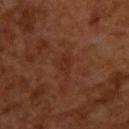Part of a total-body skin-imaging series; this lesion was reviewed on a skin check and was not flagged for biopsy. A roughly 15 mm field-of-view crop from a total-body skin photograph. This is a cross-polarized tile. A male patient, aged approximately 65. The lesion-visualizer software estimated an area of roughly 4 mm² and a shape-asymmetry score of about 0.3 (0 = symmetric). The software also gave an average lesion color of about L≈28 a*≈23 b*≈27 (CIELAB), roughly 4 lightness units darker than nearby skin, and a normalized lesion–skin contrast near 4.5. The software also gave an automated nevus-likeness rating near 0 out of 100 and a lesion-detection confidence of about 100/100.